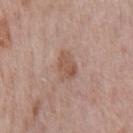Assessment: This lesion was catalogued during total-body skin photography and was not selected for biopsy. Context: A lesion tile, about 15 mm wide, cut from a 3D total-body photograph. A male subject approximately 70 years of age. An algorithmic analysis of the crop reported a within-lesion color-variation index near 2.5/10 and a peripheral color-asymmetry measure near 1. And it measured a nevus-likeness score of about 5/100. The recorded lesion diameter is about 3.5 mm. On the chest.